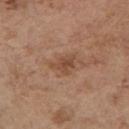- workup: imaged on a skin check; not biopsied
- location: the right upper arm
- image: ~15 mm tile from a whole-body skin photo
- lesion size: ≈3 mm
- TBP lesion metrics: an average lesion color of about L≈47 a*≈20 b*≈31 (CIELAB), about 8 CIELAB-L* units darker than the surrounding skin, and a normalized lesion–skin contrast near 6.5; a border-irregularity index near 5/10, a within-lesion color-variation index near 2.5/10, and a peripheral color-asymmetry measure near 1
- illumination: white-light
- subject: female, roughly 65 years of age